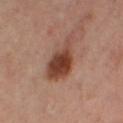<lesion>
<biopsy_status>not biopsied; imaged during a skin examination</biopsy_status>
<lesion_size>
  <long_diameter_mm_approx>5.5</long_diameter_mm_approx>
</lesion_size>
<automated_metrics>
  <border_irregularity_0_10>3.5</border_irregularity_0_10>
  <color_variation_0_10>5.0</color_variation_0_10>
  <nevus_likeness_0_100>95</nevus_likeness_0_100>
  <lesion_detection_confidence_0_100>100</lesion_detection_confidence_0_100>
</automated_metrics>
<image>
  <source>total-body photography crop</source>
  <field_of_view_mm>15</field_of_view_mm>
</image>
<site>leg</site>
<patient>
  <sex>female</sex>
  <age_approx>40</age_approx>
</patient>
<lighting>cross-polarized</lighting>
</lesion>